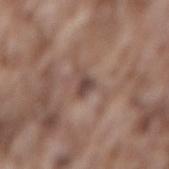The lesion was photographed on a routine skin check and not biopsied; there is no pathology result. This is a white-light tile. The lesion is on the lower back. The total-body-photography lesion software estimated a footprint of about 3.5 mm² and a shape eccentricity near 0.7. And it measured internal color variation of about 1.5 on a 0–10 scale and peripheral color asymmetry of about 0.5. Cropped from a whole-body photographic skin survey; the tile spans about 15 mm. A male subject, in their mid- to late 70s. Approximately 2.5 mm at its widest.